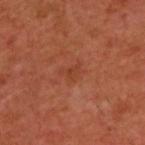The lesion was photographed on a routine skin check and not biopsied; there is no pathology result.
Measured at roughly 3 mm in maximum diameter.
Imaged with cross-polarized lighting.
A male patient aged 58 to 62.
The lesion-visualizer software estimated an average lesion color of about L≈39 a*≈27 b*≈32 (CIELAB) and a lesion–skin lightness drop of about 5. It also reported a color-variation rating of about 2/10 and peripheral color asymmetry of about 1.
Located on the upper back.
Cropped from a whole-body photographic skin survey; the tile spans about 15 mm.